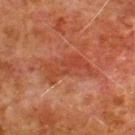Clinical impression:
The lesion was tiled from a total-body skin photograph and was not biopsied.
Clinical summary:
Captured under cross-polarized illumination. Approximately 6.5 mm at its widest. From the upper back. A male patient aged approximately 80. A roughly 15 mm field-of-view crop from a total-body skin photograph.